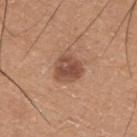Notes:
- notes — no biopsy performed (imaged during a skin exam)
- acquisition — total-body-photography crop, ~15 mm field of view
- diameter — ~3.5 mm (longest diameter)
- TBP lesion metrics — a normalized border contrast of about 8.5
- location — the upper back
- subject — male, aged 18–22
- tile lighting — white-light illumination